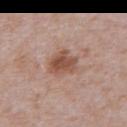  biopsy_status: not biopsied; imaged during a skin examination
  lighting: white-light
  site: abdomen
  image:
    source: total-body photography crop
    field_of_view_mm: 15
  patient:
    sex: male
    age_approx: 65